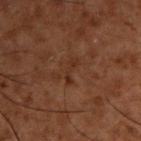The lesion was tiled from a total-body skin photograph and was not biopsied.